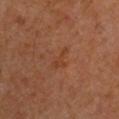Assessment: No biopsy was performed on this lesion — it was imaged during a full skin examination and was not determined to be concerning.